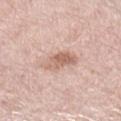Q: Was a biopsy performed?
A: no biopsy performed (imaged during a skin exam)
Q: What are the patient's age and sex?
A: female, about 65 years old
Q: How large is the lesion?
A: ≈4 mm
Q: How was this image acquired?
A: 15 mm crop, total-body photography
Q: How was the tile lit?
A: white-light illumination
Q: Lesion location?
A: the leg
Q: What did automated image analysis measure?
A: an area of roughly 7 mm², an outline eccentricity of about 0.8 (0 = round, 1 = elongated), and a shape-asymmetry score of about 0.35 (0 = symmetric); a border-irregularity rating of about 3.5/10, a within-lesion color-variation index near 5/10, and a peripheral color-asymmetry measure near 2; a nevus-likeness score of about 50/100 and a lesion-detection confidence of about 100/100